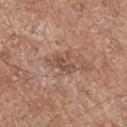The lesion was tiled from a total-body skin photograph and was not biopsied. About 3.5 mm across. An algorithmic analysis of the crop reported an area of roughly 5 mm², a shape eccentricity near 0.75, and a shape-asymmetry score of about 0.35 (0 = symmetric). The software also gave a lesion-detection confidence of about 100/100. Located on the left upper arm. The tile uses white-light illumination. A roughly 15 mm field-of-view crop from a total-body skin photograph. The subject is a male aged approximately 70.